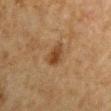| field | value |
|---|---|
| workup | catalogued during a skin exam; not biopsied |
| size | ≈3.5 mm |
| imaging modality | total-body-photography crop, ~15 mm field of view |
| body site | the arm |
| subject | male, about 50 years old |